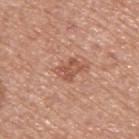No biopsy was performed on this lesion — it was imaged during a full skin examination and was not determined to be concerning. The lesion is on the upper back. Measured at roughly 3 mm in maximum diameter. A lesion tile, about 15 mm wide, cut from a 3D total-body photograph. A male subject, in their mid-50s. Imaged with white-light lighting.